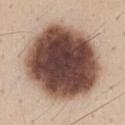Context:
From the front of the torso. Measured at roughly 10 mm in maximum diameter. Cropped from a total-body skin-imaging series; the visible field is about 15 mm. Automated image analysis of the tile measured a lesion area of about 70 mm² and a shape eccentricity near 0.45. And it measured an average lesion color of about L≈46 a*≈18 b*≈24 (CIELAB) and a lesion-to-skin contrast of about 17 (normalized; higher = more distinct). A male patient aged 33–37. This is a white-light tile.
Conclusion:
Histopathology of the biopsied lesion showed a dysplastic (Clark) nevus — a benign skin lesion.